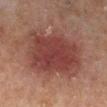Assessment:
The lesion was photographed on a routine skin check and not biopsied; there is no pathology result.
Image and clinical context:
The patient is a male aged approximately 65. The total-body-photography lesion software estimated a lesion color around L≈32 a*≈20 b*≈20 in CIELAB and about 9 CIELAB-L* units darker than the surrounding skin. And it measured a border-irregularity index near 2.5/10 and peripheral color asymmetry of about 1. Located on the left lower leg. Imaged with cross-polarized lighting. A 15 mm close-up extracted from a 3D total-body photography capture.